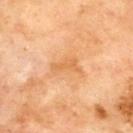From the upper back. The lesion-visualizer software estimated a lesion color around L≈64 a*≈24 b*≈44 in CIELAB and a normalized border contrast of about 5.5. The analysis additionally found an automated nevus-likeness rating near 0 out of 100 and a detector confidence of about 100 out of 100 that the crop contains a lesion. Approximately 3.5 mm at its widest. A male patient, about 70 years old. Cropped from a whole-body photographic skin survey; the tile spans about 15 mm.